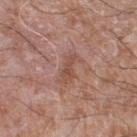{"biopsy_status": "not biopsied; imaged during a skin examination", "lesion_size": {"long_diameter_mm_approx": 3.5}, "site": "leg", "lighting": "white-light", "automated_metrics": {"border_irregularity_0_10": 5.0, "color_variation_0_10": 1.0, "peripheral_color_asymmetry": 0.5}, "image": {"source": "total-body photography crop", "field_of_view_mm": 15}, "patient": {"sex": "male", "age_approx": 60}}